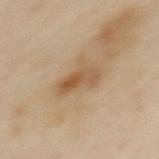Assessment:
Part of a total-body skin-imaging series; this lesion was reviewed on a skin check and was not flagged for biopsy.
Acquisition and patient details:
A 15 mm close-up extracted from a 3D total-body photography capture. From the upper back. A female patient aged 38 to 42.